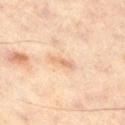The lesion was tiled from a total-body skin photograph and was not biopsied. A close-up tile cropped from a whole-body skin photograph, about 15 mm across. From the leg. A male subject, about 60 years old.